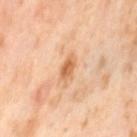Background: A lesion tile, about 15 mm wide, cut from a 3D total-body photograph. This is a cross-polarized tile. The lesion is located on the leg. The subject is a female in their mid-50s. The lesion-visualizer software estimated a mean CIELAB color near L≈67 a*≈24 b*≈41, about 11 CIELAB-L* units darker than the surrounding skin, and a normalized border contrast of about 7.5. The analysis additionally found border irregularity of about 2.5 on a 0–10 scale, internal color variation of about 3.5 on a 0–10 scale, and a peripheral color-asymmetry measure near 1.